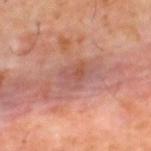| key | value |
|---|---|
| notes | catalogued during a skin exam; not biopsied |